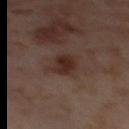No biopsy was performed on this lesion — it was imaged during a full skin examination and was not determined to be concerning. The recorded lesion diameter is about 4 mm. Cropped from a whole-body photographic skin survey; the tile spans about 15 mm. A female patient, aged approximately 55. The total-body-photography lesion software estimated an area of roughly 8.5 mm², a shape eccentricity near 0.7, and a symmetry-axis asymmetry near 0.3. And it measured border irregularity of about 3 on a 0–10 scale, a color-variation rating of about 3.5/10, and a peripheral color-asymmetry measure near 1. The analysis additionally found a classifier nevus-likeness of about 80/100 and lesion-presence confidence of about 100/100. The lesion is located on the right thigh.